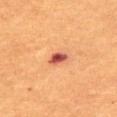The lesion was tiled from a total-body skin photograph and was not biopsied.
Captured under cross-polarized illumination.
An algorithmic analysis of the crop reported an area of roughly 4 mm² and a shape eccentricity near 0.75. The software also gave a border-irregularity rating of about 2/10, internal color variation of about 5.5 on a 0–10 scale, and peripheral color asymmetry of about 1.5. And it measured a lesion-detection confidence of about 100/100.
Approximately 2.5 mm at its widest.
This image is a 15 mm lesion crop taken from a total-body photograph.
A male subject, aged 63–67.
Located on the chest.